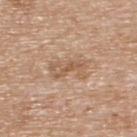Assessment: The lesion was tiled from a total-body skin photograph and was not biopsied. Context: On the upper back. A male patient, aged 58 to 62. Cropped from a total-body skin-imaging series; the visible field is about 15 mm.